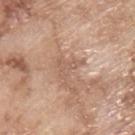biopsy_status: not biopsied; imaged during a skin examination
image:
  source: total-body photography crop
  field_of_view_mm: 15
patient:
  sex: male
  age_approx: 65
site: upper back
lesion_size:
  long_diameter_mm_approx: 3.5
lighting: white-light
automated_metrics:
  cielab_L: 58
  cielab_a: 19
  cielab_b: 29
  border_irregularity_0_10: 7.0
  color_variation_0_10: 1.0
  peripheral_color_asymmetry: 0.5